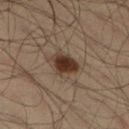<lesion>
  <biopsy_status>not biopsied; imaged during a skin examination</biopsy_status>
  <image>
    <source>total-body photography crop</source>
    <field_of_view_mm>15</field_of_view_mm>
  </image>
  <site>right lower leg</site>
  <patient>
    <sex>male</sex>
    <age_approx>35</age_approx>
  </patient>
  <automated_metrics>
    <area_mm2_approx>7.0</area_mm2_approx>
    <eccentricity>0.65</eccentricity>
    <shape_asymmetry>0.3</shape_asymmetry>
    <cielab_L>31</cielab_L>
    <cielab_a>15</cielab_a>
    <cielab_b>23</cielab_b>
    <vs_skin_contrast_norm>12.0</vs_skin_contrast_norm>
    <peripheral_color_asymmetry>1.0</peripheral_color_asymmetry>
    <nevus_likeness_0_100>100</nevus_likeness_0_100>
    <lesion_detection_confidence_0_100>100</lesion_detection_confidence_0_100>
  </automated_metrics>
  <lighting>cross-polarized</lighting>
  <lesion_size>
    <long_diameter_mm_approx>3.5</long_diameter_mm_approx>
  </lesion_size>
</lesion>